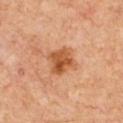Notes:
– biopsy status: catalogued during a skin exam; not biopsied
– anatomic site: the front of the torso
– image: ~15 mm crop, total-body skin-cancer survey
– patient: male, aged approximately 60
– TBP lesion metrics: a lesion area of about 8 mm², an outline eccentricity of about 0.45 (0 = round, 1 = elongated), and a shape-asymmetry score of about 0.2 (0 = symmetric); a mean CIELAB color near L≈52 a*≈26 b*≈39, about 12 CIELAB-L* units darker than the surrounding skin, and a lesion-to-skin contrast of about 9 (normalized; higher = more distinct); a classifier nevus-likeness of about 75/100